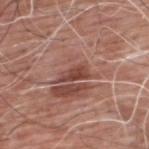workup=imaged on a skin check; not biopsied | subject=male, roughly 60 years of age | diameter=about 3.5 mm | site=the upper back | image=total-body-photography crop, ~15 mm field of view.